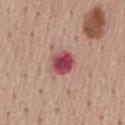Impression: No biopsy was performed on this lesion — it was imaged during a full skin examination and was not determined to be concerning. Acquisition and patient details: The patient is a male aged 53 to 57. Longest diameter approximately 3 mm. Captured under white-light illumination. The lesion is on the mid back. A lesion tile, about 15 mm wide, cut from a 3D total-body photograph.